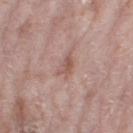biopsy_status: not biopsied; imaged during a skin examination
automated_metrics:
  border_irregularity_0_10: 4.0
  color_variation_0_10: 3.0
  peripheral_color_asymmetry: 1.0
  nevus_likeness_0_100: 0
  lesion_detection_confidence_0_100: 100
site: right thigh
image:
  source: total-body photography crop
  field_of_view_mm: 15
lighting: white-light
patient:
  sex: female
  age_approx: 70
lesion_size:
  long_diameter_mm_approx: 3.0